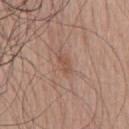Findings:
• workup · catalogued during a skin exam; not biopsied
• location · the chest
• lesion diameter · about 2.5 mm
• tile lighting · white-light
• patient · male, approximately 70 years of age
• image · ~15 mm crop, total-body skin-cancer survey
• automated metrics · an outline eccentricity of about 0.9 (0 = round, 1 = elongated); an automated nevus-likeness rating near 0 out of 100 and a detector confidence of about 100 out of 100 that the crop contains a lesion